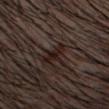Recorded during total-body skin imaging; not selected for excision or biopsy. A male patient in their mid-30s. A roughly 15 mm field-of-view crop from a total-body skin photograph. The lesion is located on the head or neck.